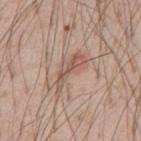The lesion was photographed on a routine skin check and not biopsied; there is no pathology result.
From the abdomen.
The lesion-visualizer software estimated an automated nevus-likeness rating near 0 out of 100 and lesion-presence confidence of about 70/100.
The patient is a male aged approximately 55.
Captured under white-light illumination.
Approximately 5.5 mm at its widest.
A lesion tile, about 15 mm wide, cut from a 3D total-body photograph.